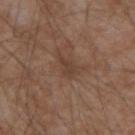The lesion was tiled from a total-body skin photograph and was not biopsied. Automated tile analysis of the lesion measured a lesion color around L≈40 a*≈17 b*≈25 in CIELAB, about 7 CIELAB-L* units darker than the surrounding skin, and a normalized lesion–skin contrast near 6. And it measured border irregularity of about 4 on a 0–10 scale, a color-variation rating of about 0.5/10, and peripheral color asymmetry of about 0. The software also gave an automated nevus-likeness rating near 0 out of 100 and a detector confidence of about 100 out of 100 that the crop contains a lesion. The lesion's longest dimension is about 2.5 mm. The lesion is on the right forearm. A male patient approximately 75 years of age. A 15 mm crop from a total-body photograph taken for skin-cancer surveillance.